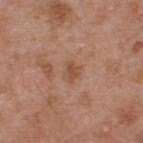Captured during whole-body skin photography for melanoma surveillance; the lesion was not biopsied. A close-up tile cropped from a whole-body skin photograph, about 15 mm across. This is a white-light tile. The lesion-visualizer software estimated a mean CIELAB color near L≈51 a*≈22 b*≈30, about 7 CIELAB-L* units darker than the surrounding skin, and a lesion-to-skin contrast of about 5.5 (normalized; higher = more distinct). About 2.5 mm across. The lesion is located on the upper back. The patient is a male roughly 55 years of age.